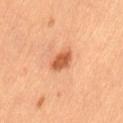Assessment: The lesion was photographed on a routine skin check and not biopsied; there is no pathology result. Clinical summary: Imaged with cross-polarized lighting. A female subject, in their 60s. Cropped from a total-body skin-imaging series; the visible field is about 15 mm. Approximately 3 mm at its widest. The lesion is on the lower back.